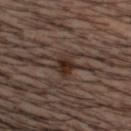No biopsy was performed on this lesion — it was imaged during a full skin examination and was not determined to be concerning.
Measured at roughly 3 mm in maximum diameter.
A lesion tile, about 15 mm wide, cut from a 3D total-body photograph.
The patient is a male aged 33 to 37.
Located on the head or neck.
The tile uses cross-polarized illumination.